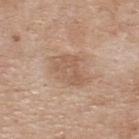Recorded during total-body skin imaging; not selected for excision or biopsy. The lesion's longest dimension is about 5 mm. The patient is a male aged 73 to 77. The lesion is on the upper back. A 15 mm close-up tile from a total-body photography series done for melanoma screening.